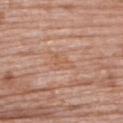Q: Is there a histopathology result?
A: total-body-photography surveillance lesion; no biopsy
Q: Automated lesion metrics?
A: a lesion area of about 4 mm², an outline eccentricity of about 0.85 (0 = round, 1 = elongated), and a symmetry-axis asymmetry near 0.45; a mean CIELAB color near L≈58 a*≈21 b*≈32, about 5 CIELAB-L* units darker than the surrounding skin, and a normalized border contrast of about 5; a detector confidence of about 95 out of 100 that the crop contains a lesion
Q: Where on the body is the lesion?
A: the upper back
Q: What is the imaging modality?
A: ~15 mm tile from a whole-body skin photo
Q: What are the patient's age and sex?
A: female, about 70 years old